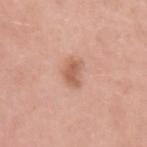biopsy status = catalogued during a skin exam; not biopsied
size = ~3 mm (longest diameter)
illumination = white-light
body site = the left upper arm
patient = female, in their mid-40s
automated lesion analysis = a footprint of about 5.5 mm² and a shape eccentricity near 0.65; a color-variation rating of about 2.5/10 and radial color variation of about 1; a classifier nevus-likeness of about 85/100 and a detector confidence of about 100 out of 100 that the crop contains a lesion
image source = 15 mm crop, total-body photography